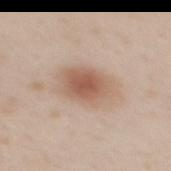Impression:
No biopsy was performed on this lesion — it was imaged during a full skin examination and was not determined to be concerning.
Acquisition and patient details:
Automated tile analysis of the lesion measured a footprint of about 15 mm², a shape eccentricity near 0.75, and a shape-asymmetry score of about 0.15 (0 = symmetric). The analysis additionally found an average lesion color of about L≈59 a*≈18 b*≈28 (CIELAB), roughly 11 lightness units darker than nearby skin, and a lesion-to-skin contrast of about 7.5 (normalized; higher = more distinct). The analysis additionally found a border-irregularity index near 2/10 and peripheral color asymmetry of about 1. And it measured an automated nevus-likeness rating near 95 out of 100 and a detector confidence of about 100 out of 100 that the crop contains a lesion. A 15 mm close-up tile from a total-body photography series done for melanoma screening. A female subject, roughly 30 years of age. On the mid back.